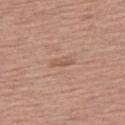Case summary:
- biopsy status: total-body-photography surveillance lesion; no biopsy
- anatomic site: the right thigh
- tile lighting: white-light illumination
- image: total-body-photography crop, ~15 mm field of view
- size: ~3 mm (longest diameter)
- subject: female, aged approximately 60
- automated lesion analysis: about 7 CIELAB-L* units darker than the surrounding skin and a normalized lesion–skin contrast near 5; a within-lesion color-variation index near 0.5/10 and a peripheral color-asymmetry measure near 0; an automated nevus-likeness rating near 0 out of 100 and lesion-presence confidence of about 100/100